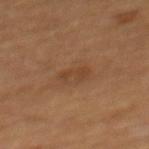Captured during whole-body skin photography for melanoma surveillance; the lesion was not biopsied.
The lesion's longest dimension is about 3 mm.
A region of skin cropped from a whole-body photographic capture, roughly 15 mm wide.
Imaged with cross-polarized lighting.
The lesion is on the mid back.
An algorithmic analysis of the crop reported a border-irregularity rating of about 4.5/10, internal color variation of about 0.5 on a 0–10 scale, and a peripheral color-asymmetry measure near 0. And it measured a classifier nevus-likeness of about 0/100 and lesion-presence confidence of about 100/100.
A male subject aged 58 to 62.